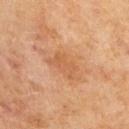About 6.5 mm across. A male patient aged 63 to 67. The lesion-visualizer software estimated an average lesion color of about L≈59 a*≈22 b*≈37 (CIELAB) and a lesion-to-skin contrast of about 4.5 (normalized; higher = more distinct). The analysis additionally found a classifier nevus-likeness of about 0/100 and lesion-presence confidence of about 100/100. This image is a 15 mm lesion crop taken from a total-body photograph. This is a cross-polarized tile.